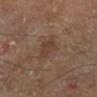Assessment:
No biopsy was performed on this lesion — it was imaged during a full skin examination and was not determined to be concerning.
Clinical summary:
The lesion-visualizer software estimated an average lesion color of about L≈38 a*≈17 b*≈24 (CIELAB) and a normalized lesion–skin contrast near 6. The software also gave border irregularity of about 3.5 on a 0–10 scale, internal color variation of about 2 on a 0–10 scale, and a peripheral color-asymmetry measure near 0.5. A male subject, aged 68 to 72. A 15 mm close-up tile from a total-body photography series done for melanoma screening. The lesion is on the right lower leg.